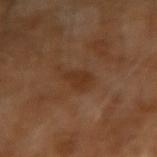biopsy_status: not biopsied; imaged during a skin examination
lesion_size:
  long_diameter_mm_approx: 2.5
image:
  source: total-body photography crop
  field_of_view_mm: 15
patient:
  sex: male
  age_approx: 65